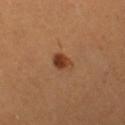Captured during whole-body skin photography for melanoma surveillance; the lesion was not biopsied.
From the leg.
A female patient, roughly 25 years of age.
The recorded lesion diameter is about 3 mm.
The tile uses cross-polarized illumination.
This image is a 15 mm lesion crop taken from a total-body photograph.